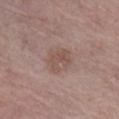{
  "biopsy_status": "not biopsied; imaged during a skin examination",
  "image": {
    "source": "total-body photography crop",
    "field_of_view_mm": 15
  },
  "site": "right lower leg",
  "automated_metrics": {
    "cielab_L": 51,
    "cielab_a": 17,
    "cielab_b": 24,
    "vs_skin_darker_L": 7.0,
    "color_variation_0_10": 2.0,
    "peripheral_color_asymmetry": 0.5
  },
  "lesion_size": {
    "long_diameter_mm_approx": 3.0
  },
  "lighting": "white-light",
  "patient": {
    "sex": "female",
    "age_approx": 65
  }
}